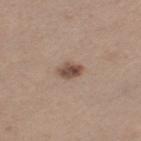{"biopsy_status": "not biopsied; imaged during a skin examination", "lesion_size": {"long_diameter_mm_approx": 2.5}, "patient": {"sex": "female", "age_approx": 45}, "image": {"source": "total-body photography crop", "field_of_view_mm": 15}, "lighting": "white-light", "site": "left thigh", "automated_metrics": {"color_variation_0_10": 4.5, "nevus_likeness_0_100": 95, "lesion_detection_confidence_0_100": 100}}